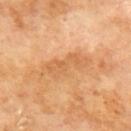Impression: Captured during whole-body skin photography for melanoma surveillance; the lesion was not biopsied. Image and clinical context: Measured at roughly 6 mm in maximum diameter. Cropped from a total-body skin-imaging series; the visible field is about 15 mm. On the mid back. A male subject, aged 68 to 72.